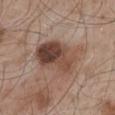{
  "biopsy_status": "not biopsied; imaged during a skin examination",
  "site": "back",
  "image": {
    "source": "total-body photography crop",
    "field_of_view_mm": 15
  },
  "lighting": "white-light",
  "patient": {
    "sex": "male",
    "age_approx": 55
  },
  "automated_metrics": {
    "area_mm2_approx": 21.0,
    "shape_asymmetry": 0.15,
    "border_irregularity_0_10": 2.5,
    "color_variation_0_10": 9.5,
    "peripheral_color_asymmetry": 4.5
  }
}